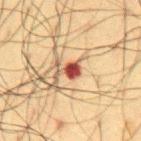| field | value |
|---|---|
| lesion diameter | ≈3 mm |
| acquisition | ~15 mm tile from a whole-body skin photo |
| tile lighting | cross-polarized |
| subject | male, aged approximately 60 |
| site | the chest |
| TBP lesion metrics | a footprint of about 3.5 mm² and an outline eccentricity of about 0.85 (0 = round, 1 = elongated); a border-irregularity rating of about 5/10, internal color variation of about 2.5 on a 0–10 scale, and a peripheral color-asymmetry measure near 1; a detector confidence of about 100 out of 100 that the crop contains a lesion |